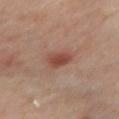Part of a total-body skin-imaging series; this lesion was reviewed on a skin check and was not flagged for biopsy. The patient is a female about 40 years old. The lesion is on the left arm. Approximately 2.5 mm at its widest. Imaged with cross-polarized lighting. A 15 mm close-up tile from a total-body photography series done for melanoma screening.